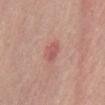Part of a total-body skin-imaging series; this lesion was reviewed on a skin check and was not flagged for biopsy.
A region of skin cropped from a whole-body photographic capture, roughly 15 mm wide.
A male subject about 65 years old.
Approximately 3 mm at its widest.
From the abdomen.